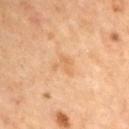Clinical impression:
Imaged during a routine full-body skin examination; the lesion was not biopsied and no histopathology is available.
Image and clinical context:
The lesion is on the arm. The patient is a male aged around 70. A roughly 15 mm field-of-view crop from a total-body skin photograph. The lesion's longest dimension is about 2.5 mm. Automated image analysis of the tile measured a lesion area of about 4 mm², an outline eccentricity of about 0.55 (0 = round, 1 = elongated), and a shape-asymmetry score of about 0.55 (0 = symmetric). And it measured roughly 5 lightness units darker than nearby skin. And it measured border irregularity of about 5.5 on a 0–10 scale, a color-variation rating of about 1.5/10, and peripheral color asymmetry of about 0.5. The tile uses cross-polarized illumination.